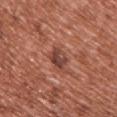This lesion was catalogued during total-body skin photography and was not selected for biopsy.
The lesion is on the upper back.
The lesion-visualizer software estimated a footprint of about 5 mm², an outline eccentricity of about 0.75 (0 = round, 1 = elongated), and a symmetry-axis asymmetry near 0.25. And it measured a lesion color around L≈43 a*≈25 b*≈27 in CIELAB, a lesion–skin lightness drop of about 11, and a lesion-to-skin contrast of about 8.5 (normalized; higher = more distinct). It also reported border irregularity of about 3 on a 0–10 scale, a color-variation rating of about 3.5/10, and radial color variation of about 1. And it measured a nevus-likeness score of about 5/100 and lesion-presence confidence of about 100/100.
A female patient about 40 years old.
Captured under white-light illumination.
A region of skin cropped from a whole-body photographic capture, roughly 15 mm wide.
Longest diameter approximately 3 mm.